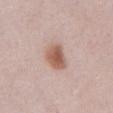This image is a 15 mm lesion crop taken from a total-body photograph.
The patient is a female roughly 60 years of age.
Located on the abdomen.
Imaged with white-light lighting.